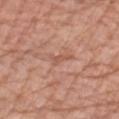• workup: no biopsy performed (imaged during a skin exam)
• anatomic site: the left forearm
• subject: male, aged around 75
• automated lesion analysis: two-axis asymmetry of about 0.6; an automated nevus-likeness rating near 0 out of 100 and lesion-presence confidence of about 90/100
• image: total-body-photography crop, ~15 mm field of view
• lesion diameter: ≈2.5 mm
• tile lighting: white-light illumination